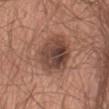Impression: The lesion was photographed on a routine skin check and not biopsied; there is no pathology result. Background: A 15 mm close-up extracted from a 3D total-body photography capture. Approximately 6 mm at its widest. This is a white-light tile. The lesion is located on the abdomen. A male subject, approximately 65 years of age.